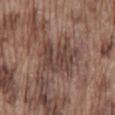Imaged during a routine full-body skin examination; the lesion was not biopsied and no histopathology is available.
Imaged with white-light lighting.
Longest diameter approximately 5 mm.
The lesion-visualizer software estimated an area of roughly 12 mm² and an eccentricity of roughly 0.7. The analysis additionally found an automated nevus-likeness rating near 0 out of 100.
From the mid back.
A roughly 15 mm field-of-view crop from a total-body skin photograph.
A male patient aged 73 to 77.